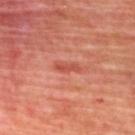Imaged during a routine full-body skin examination; the lesion was not biopsied and no histopathology is available.
A male patient approximately 70 years of age.
The recorded lesion diameter is about 3 mm.
The lesion is located on the upper back.
A 15 mm crop from a total-body photograph taken for skin-cancer surveillance.
The tile uses cross-polarized illumination.
The lesion-visualizer software estimated an area of roughly 3 mm² and a symmetry-axis asymmetry near 0.3. It also reported an automated nevus-likeness rating near 0 out of 100 and a lesion-detection confidence of about 90/100.